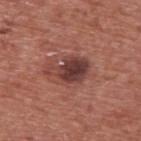Notes:
* follow-up — no biopsy performed (imaged during a skin exam)
* lighting — white-light
* imaging modality — ~15 mm crop, total-body skin-cancer survey
* lesion size — about 5 mm
* subject — male, aged 73 to 77
* site — the upper back